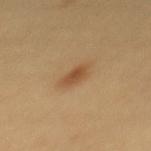Impression:
This lesion was catalogued during total-body skin photography and was not selected for biopsy.
Clinical summary:
Located on the mid back. This image is a 15 mm lesion crop taken from a total-body photograph. Captured under cross-polarized illumination. A female subject aged 23–27. About 3 mm across.